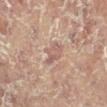patient:
  sex: female
  age_approx: 80
lesion_size:
  long_diameter_mm_approx: 3.5
site: leg
image:
  source: total-body photography crop
  field_of_view_mm: 15